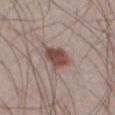Case summary:
– notes — imaged on a skin check; not biopsied
– lesion size — ~3.5 mm (longest diameter)
– image — total-body-photography crop, ~15 mm field of view
– subject — male, about 50 years old
– image-analysis metrics — an area of roughly 7.5 mm²
– site — the left thigh